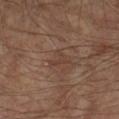* workup: catalogued during a skin exam; not biopsied
* size: ≈3 mm
* location: the left forearm
* subject: male, in their mid-60s
* illumination: cross-polarized illumination
* acquisition: total-body-photography crop, ~15 mm field of view